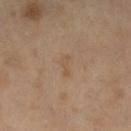{"biopsy_status": "not biopsied; imaged during a skin examination", "image": {"source": "total-body photography crop", "field_of_view_mm": 15}, "site": "left thigh", "patient": {"sex": "female", "age_approx": 55}}